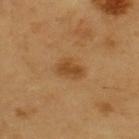Captured during whole-body skin photography for melanoma surveillance; the lesion was not biopsied.
On the upper back.
This is a cross-polarized tile.
A male subject aged 58–62.
Longest diameter approximately 3 mm.
A 15 mm close-up tile from a total-body photography series done for melanoma screening.